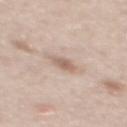biopsy_status: not biopsied; imaged during a skin examination
lighting: white-light
patient:
  sex: male
  age_approx: 45
image:
  source: total-body photography crop
  field_of_view_mm: 15
site: back